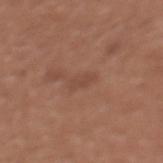Recorded during total-body skin imaging; not selected for excision or biopsy.
A female patient approximately 35 years of age.
The lesion-visualizer software estimated an average lesion color of about L≈46 a*≈21 b*≈28 (CIELAB), roughly 6 lightness units darker than nearby skin, and a normalized border contrast of about 4.5. And it measured a border-irregularity rating of about 3/10, internal color variation of about 0.5 on a 0–10 scale, and radial color variation of about 0. And it measured lesion-presence confidence of about 100/100.
Located on the chest.
Cropped from a total-body skin-imaging series; the visible field is about 15 mm.